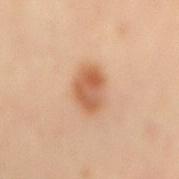Clinical impression:
No biopsy was performed on this lesion — it was imaged during a full skin examination and was not determined to be concerning.
Clinical summary:
An algorithmic analysis of the crop reported an outline eccentricity of about 0.8 (0 = round, 1 = elongated) and two-axis asymmetry of about 0.15. It also reported an average lesion color of about L≈60 a*≈23 b*≈36 (CIELAB) and roughly 12 lightness units darker than nearby skin. A 15 mm crop from a total-body photograph taken for skin-cancer surveillance. Measured at roughly 4.5 mm in maximum diameter. A male patient, in their mid-60s. The lesion is on the mid back.